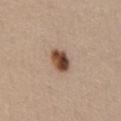Part of a total-body skin-imaging series; this lesion was reviewed on a skin check and was not flagged for biopsy. A female patient, aged approximately 45. On the chest. A close-up tile cropped from a whole-body skin photograph, about 15 mm across. This is a white-light tile. Measured at roughly 3.5 mm in maximum diameter.